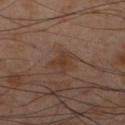Impression:
Imaged during a routine full-body skin examination; the lesion was not biopsied and no histopathology is available.
Clinical summary:
Longest diameter approximately 3 mm. The lesion is on the right lower leg. The total-body-photography lesion software estimated a lesion color around L≈36 a*≈18 b*≈26 in CIELAB, a lesion–skin lightness drop of about 6, and a normalized lesion–skin contrast near 6.5. The patient is a male aged 53–57. Cropped from a whole-body photographic skin survey; the tile spans about 15 mm.